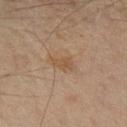image-analysis metrics: a footprint of about 4.5 mm², a shape eccentricity near 0.85, and two-axis asymmetry of about 0.35; an average lesion color of about L≈43 a*≈14 b*≈28 (CIELAB) and a lesion-to-skin contrast of about 5.5 (normalized; higher = more distinct); a nevus-likeness score of about 0/100 and a lesion-detection confidence of about 100/100 | anatomic site: the arm | lesion diameter: ~3 mm (longest diameter) | illumination: cross-polarized | image: 15 mm crop, total-body photography | patient: male, about 45 years old.